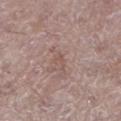tile lighting = white-light | automated lesion analysis = an average lesion color of about L≈53 a*≈18 b*≈23 (CIELAB), about 6 CIELAB-L* units darker than the surrounding skin, and a normalized lesion–skin contrast near 4.5; a classifier nevus-likeness of about 0/100 | lesion diameter = ≈2.5 mm | image source = 15 mm crop, total-body photography | patient = female, approximately 65 years of age | location = the left lower leg.